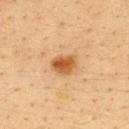follow-up = catalogued during a skin exam; not biopsied
automated metrics = a lesion color around L≈49 a*≈22 b*≈38 in CIELAB and about 12 CIELAB-L* units darker than the surrounding skin; a color-variation rating of about 4/10
diameter = about 3 mm
body site = the upper back
acquisition = ~15 mm crop, total-body skin-cancer survey
patient = female, aged 38–42